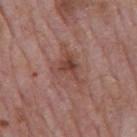Recorded during total-body skin imaging; not selected for excision or biopsy. A male patient, in their mid-70s. This is a white-light tile. A 15 mm close-up extracted from a 3D total-body photography capture. About 4 mm across. Automated tile analysis of the lesion measured an average lesion color of about L≈45 a*≈22 b*≈25 (CIELAB) and about 9 CIELAB-L* units darker than the surrounding skin. And it measured a border-irregularity index near 5.5/10 and a within-lesion color-variation index near 5.5/10. The lesion is on the mid back.